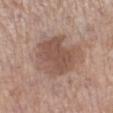biopsy status = imaged on a skin check; not biopsied | patient = female, approximately 50 years of age | lesion diameter = ≈6.5 mm | image = total-body-photography crop, ~15 mm field of view | anatomic site = the left lower leg | lighting = white-light.